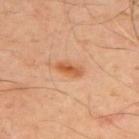Recorded during total-body skin imaging; not selected for excision or biopsy. The patient is a male aged 48 to 52. Captured under cross-polarized illumination. Automated image analysis of the tile measured a mean CIELAB color near L≈58 a*≈26 b*≈40, about 10 CIELAB-L* units darker than the surrounding skin, and a normalized border contrast of about 8. It also reported a nevus-likeness score of about 90/100. From the upper back. A region of skin cropped from a whole-body photographic capture, roughly 15 mm wide.